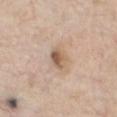Imaged during a routine full-body skin examination; the lesion was not biopsied and no histopathology is available.
Cropped from a whole-body photographic skin survey; the tile spans about 15 mm.
A male subject, aged around 60.
On the chest.